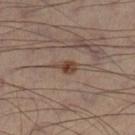notes: catalogued during a skin exam; not biopsied | site: the left leg | lesion diameter: ≈2.5 mm | patient: male, about 50 years old | image: total-body-photography crop, ~15 mm field of view | lighting: cross-polarized illumination.